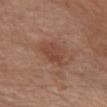No biopsy was performed on this lesion — it was imaged during a full skin examination and was not determined to be concerning.
This is a white-light tile.
Automated tile analysis of the lesion measured a footprint of about 8 mm², an eccentricity of roughly 0.75, and two-axis asymmetry of about 0.25. It also reported a lesion color around L≈45 a*≈22 b*≈28 in CIELAB and about 8 CIELAB-L* units darker than the surrounding skin. The software also gave a color-variation rating of about 2.5/10.
Cropped from a whole-body photographic skin survey; the tile spans about 15 mm.
The patient is a female about 70 years old.
The lesion is on the chest.
The lesion's longest dimension is about 4 mm.